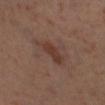Part of a total-body skin-imaging series; this lesion was reviewed on a skin check and was not flagged for biopsy. Imaged with cross-polarized lighting. The subject is a female in their 50s. From the right lower leg. Approximately 3 mm at its widest. A lesion tile, about 15 mm wide, cut from a 3D total-body photograph. The total-body-photography lesion software estimated an outline eccentricity of about 0.85 (0 = round, 1 = elongated) and a symmetry-axis asymmetry near 0.25. The software also gave a lesion color around L≈34 a*≈19 b*≈24 in CIELAB, a lesion–skin lightness drop of about 7, and a lesion-to-skin contrast of about 7 (normalized; higher = more distinct).